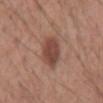The patient is a male about 55 years old. Automated tile analysis of the lesion measured a footprint of about 10 mm², an outline eccentricity of about 0.8 (0 = round, 1 = elongated), and two-axis asymmetry of about 0.2. It also reported a lesion color around L≈45 a*≈21 b*≈26 in CIELAB. And it measured a lesion-detection confidence of about 100/100. The tile uses white-light illumination. A 15 mm close-up extracted from a 3D total-body photography capture. Located on the mid back. The recorded lesion diameter is about 4.5 mm.